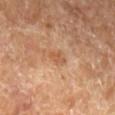Recorded during total-body skin imaging; not selected for excision or biopsy.
A female subject aged 68 to 72.
The lesion is located on the left lower leg.
The lesion-visualizer software estimated a mean CIELAB color near L≈53 a*≈23 b*≈35, roughly 8 lightness units darker than nearby skin, and a lesion-to-skin contrast of about 6 (normalized; higher = more distinct). And it measured a border-irregularity rating of about 3/10, a color-variation rating of about 0/10, and a peripheral color-asymmetry measure near 0.
Imaged with cross-polarized lighting.
Cropped from a whole-body photographic skin survey; the tile spans about 15 mm.
Measured at roughly 2.5 mm in maximum diameter.